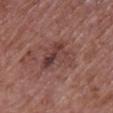size — about 4.5 mm | illumination — white-light | automated metrics — a lesion color around L≈41 a*≈21 b*≈22 in CIELAB, roughly 8 lightness units darker than nearby skin, and a normalized border contrast of about 6.5; a classifier nevus-likeness of about 5/100 and a lesion-detection confidence of about 100/100 | imaging modality — ~15 mm tile from a whole-body skin photo | site — the right upper arm | subject — female, aged approximately 70.